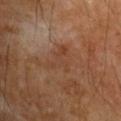Notes:
- follow-up: total-body-photography surveillance lesion; no biopsy
- location: the right upper arm
- lesion size: ≈4 mm
- illumination: cross-polarized
- automated metrics: an area of roughly 7 mm²; an average lesion color of about L≈39 a*≈19 b*≈29 (CIELAB), roughly 5 lightness units darker than nearby skin, and a lesion-to-skin contrast of about 5 (normalized; higher = more distinct); a classifier nevus-likeness of about 0/100 and lesion-presence confidence of about 100/100
- patient: male, aged 53 to 57
- imaging modality: ~15 mm tile from a whole-body skin photo